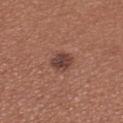Image and clinical context:
The tile uses white-light illumination. Automated image analysis of the tile measured a mean CIELAB color near L≈43 a*≈21 b*≈25 and about 10 CIELAB-L* units darker than the surrounding skin. Located on the upper back. Longest diameter approximately 3 mm. A roughly 15 mm field-of-view crop from a total-body skin photograph. A female subject approximately 25 years of age.